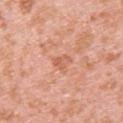Background: Located on the upper back. This is a white-light tile. A female patient aged 38 to 42. An algorithmic analysis of the crop reported a footprint of about 3 mm², a shape eccentricity near 0.8, and two-axis asymmetry of about 0.35. The analysis additionally found a lesion color around L≈61 a*≈27 b*≈35 in CIELAB, a lesion–skin lightness drop of about 8, and a lesion-to-skin contrast of about 5.5 (normalized; higher = more distinct). The software also gave lesion-presence confidence of about 100/100. Cropped from a whole-body photographic skin survey; the tile spans about 15 mm. The lesion's longest dimension is about 2.5 mm.